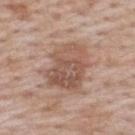Findings:
* workup — catalogued during a skin exam; not biopsied
* lesion size — ~6 mm (longest diameter)
* patient — male, aged 63–67
* body site — the upper back
* imaging modality — ~15 mm tile from a whole-body skin photo
* illumination — white-light